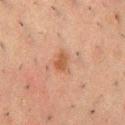patient — male, aged 48 to 52 | anatomic site — the chest | tile lighting — cross-polarized illumination | size — ≈3 mm | imaging modality — total-body-photography crop, ~15 mm field of view | automated metrics — a border-irregularity rating of about 3/10, internal color variation of about 2 on a 0–10 scale, and radial color variation of about 0.5; an automated nevus-likeness rating near 50 out of 100.